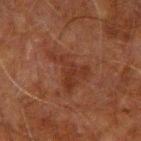<tbp_lesion>
  <biopsy_status>not biopsied; imaged during a skin examination</biopsy_status>
  <site>left arm</site>
  <patient>
    <sex>male</sex>
    <age_approx>60</age_approx>
  </patient>
  <image>
    <source>total-body photography crop</source>
    <field_of_view_mm>15</field_of_view_mm>
  </image>
  <lesion_size>
    <long_diameter_mm_approx>5.5</long_diameter_mm_approx>
  </lesion_size>
  <lighting>cross-polarized</lighting>
</tbp_lesion>